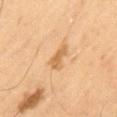| feature | finding |
|---|---|
| workup | total-body-photography surveillance lesion; no biopsy |
| patient | male, in their mid- to late 60s |
| imaging modality | ~15 mm tile from a whole-body skin photo |